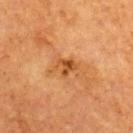Assessment: This lesion was catalogued during total-body skin photography and was not selected for biopsy. Clinical summary: Located on the back. Captured under cross-polarized illumination. Longest diameter approximately 3 mm. A close-up tile cropped from a whole-body skin photograph, about 15 mm across. The patient is a female aged 53 to 57.